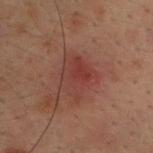{"biopsy_status": "not biopsied; imaged during a skin examination", "image": {"source": "total-body photography crop", "field_of_view_mm": 15}, "lighting": "cross-polarized", "automated_metrics": {"shape_asymmetry": 0.25, "cielab_L": 31, "cielab_a": 21, "cielab_b": 21, "vs_skin_darker_L": 6.0, "vs_skin_contrast_norm": 6.0}, "lesion_size": {"long_diameter_mm_approx": 4.5}, "site": "upper back", "patient": {"sex": "male", "age_approx": 30}}